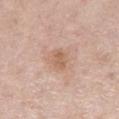Recorded during total-body skin imaging; not selected for excision or biopsy.